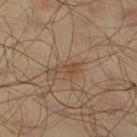No biopsy was performed on this lesion — it was imaged during a full skin examination and was not determined to be concerning.
A 15 mm crop from a total-body photograph taken for skin-cancer surveillance.
A male subject, roughly 65 years of age.
This is a cross-polarized tile.
Longest diameter approximately 3 mm.
The lesion is on the right lower leg.